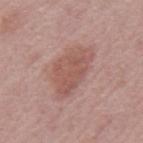– notes · total-body-photography surveillance lesion; no biopsy
– patient · male, approximately 65 years of age
– imaging modality · 15 mm crop, total-body photography
– tile lighting · white-light illumination
– size · ≈6 mm
– location · the mid back